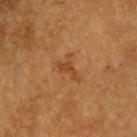Clinical impression:
Part of a total-body skin-imaging series; this lesion was reviewed on a skin check and was not flagged for biopsy.
Context:
The subject is a male roughly 75 years of age. A 15 mm close-up tile from a total-body photography series done for melanoma screening. Imaged with cross-polarized lighting. About 3 mm across. The lesion is located on the upper back.